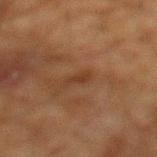| field | value |
|---|---|
| follow-up | no biopsy performed (imaged during a skin exam) |
| size | ≈2.5 mm |
| image-analysis metrics | a lesion color around L≈28 a*≈17 b*≈26 in CIELAB, roughly 5 lightness units darker than nearby skin, and a normalized border contrast of about 5.5; an automated nevus-likeness rating near 0 out of 100 and lesion-presence confidence of about 100/100 |
| acquisition | ~15 mm crop, total-body skin-cancer survey |
| anatomic site | the mid back |
| subject | male, aged 58 to 62 |
| lighting | cross-polarized illumination |